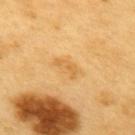| feature | finding |
|---|---|
| biopsy status | imaged on a skin check; not biopsied |
| subject | male, approximately 60 years of age |
| body site | the upper back |
| image source | ~15 mm tile from a whole-body skin photo |